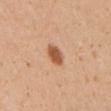No biopsy was performed on this lesion — it was imaged during a full skin examination and was not determined to be concerning. Located on the right upper arm. The subject is a female aged approximately 30. Measured at roughly 3 mm in maximum diameter. A lesion tile, about 15 mm wide, cut from a 3D total-body photograph. Imaged with white-light lighting. An algorithmic analysis of the crop reported a footprint of about 5 mm². And it measured a mean CIELAB color near L≈56 a*≈24 b*≈37, about 14 CIELAB-L* units darker than the surrounding skin, and a lesion-to-skin contrast of about 9 (normalized; higher = more distinct). It also reported radial color variation of about 1. The software also gave a classifier nevus-likeness of about 100/100.